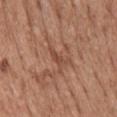TBP lesion metrics: border irregularity of about 3.5 on a 0–10 scale, a within-lesion color-variation index near 0/10, and peripheral color asymmetry of about 0; an automated nevus-likeness rating near 0 out of 100 and lesion-presence confidence of about 70/100
anatomic site: the mid back
tile lighting: white-light
acquisition: total-body-photography crop, ~15 mm field of view
subject: male, aged 58–62